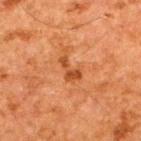This lesion was catalogued during total-body skin photography and was not selected for biopsy.
Captured under cross-polarized illumination.
Located on the upper back.
The recorded lesion diameter is about 3 mm.
A male subject, aged 58–62.
Cropped from a total-body skin-imaging series; the visible field is about 15 mm.